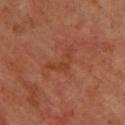{
  "biopsy_status": "not biopsied; imaged during a skin examination",
  "site": "chest",
  "image": {
    "source": "total-body photography crop",
    "field_of_view_mm": 15
  },
  "patient": {
    "sex": "male",
    "age_approx": 65
  }
}